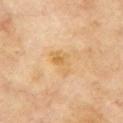Clinical impression: Recorded during total-body skin imaging; not selected for excision or biopsy. Background: Cropped from a total-body skin-imaging series; the visible field is about 15 mm. The lesion is located on the chest. Captured under cross-polarized illumination. The subject is a male aged 68–72. Measured at roughly 4 mm in maximum diameter.